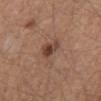Clinical impression: The lesion was tiled from a total-body skin photograph and was not biopsied. Clinical summary: A 15 mm close-up tile from a total-body photography series done for melanoma screening. The tile uses white-light illumination. Automated image analysis of the tile measured an area of roughly 7 mm², an outline eccentricity of about 0.75 (0 = round, 1 = elongated), and a shape-asymmetry score of about 0.45 (0 = symmetric). The software also gave an average lesion color of about L≈44 a*≈19 b*≈27 (CIELAB) and a lesion–skin lightness drop of about 9. The analysis additionally found lesion-presence confidence of about 100/100. On the front of the torso. A male subject, aged around 65.